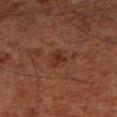The lesion was photographed on a routine skin check and not biopsied; there is no pathology result. This is a cross-polarized tile. The total-body-photography lesion software estimated an area of roughly 3.5 mm², an outline eccentricity of about 0.8 (0 = round, 1 = elongated), and a shape-asymmetry score of about 0.3 (0 = symmetric). And it measured a lesion–skin lightness drop of about 7 and a normalized lesion–skin contrast near 7. It also reported a color-variation rating of about 1.5/10 and radial color variation of about 0.5. The lesion is on the leg. A lesion tile, about 15 mm wide, cut from a 3D total-body photograph. Approximately 2.5 mm at its widest. A male subject, approximately 70 years of age.